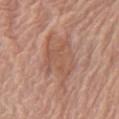<tbp_lesion>
  <biopsy_status>not biopsied; imaged during a skin examination</biopsy_status>
  <site>chest</site>
  <patient>
    <sex>male</sex>
    <age_approx>80</age_approx>
  </patient>
  <image>
    <source>total-body photography crop</source>
    <field_of_view_mm>15</field_of_view_mm>
  </image>
</tbp_lesion>